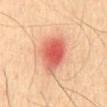Imaged during a routine full-body skin examination; the lesion was not biopsied and no histopathology is available. Imaged with cross-polarized lighting. This image is a 15 mm lesion crop taken from a total-body photograph. An algorithmic analysis of the crop reported an automated nevus-likeness rating near 25 out of 100 and a detector confidence of about 100 out of 100 that the crop contains a lesion. The subject is a male aged approximately 60. From the mid back.